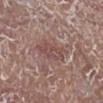Impression: This lesion was catalogued during total-body skin photography and was not selected for biopsy. Acquisition and patient details: Automated tile analysis of the lesion measured border irregularity of about 2.5 on a 0–10 scale and a color-variation rating of about 3.5/10. The lesion is on the right lower leg. A male patient aged 78 to 82. Imaged with white-light lighting. Cropped from a total-body skin-imaging series; the visible field is about 15 mm.